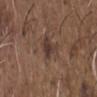follow-up: imaged on a skin check; not biopsied | subject: male, aged approximately 50 | lesion diameter: ~3 mm (longest diameter) | lighting: white-light illumination | image: 15 mm crop, total-body photography | automated metrics: a footprint of about 4.5 mm², an outline eccentricity of about 0.5 (0 = round, 1 = elongated), and a symmetry-axis asymmetry near 0.35; a border-irregularity index near 3.5/10, a color-variation rating of about 3.5/10, and radial color variation of about 1; a classifier nevus-likeness of about 5/100 and a lesion-detection confidence of about 100/100 | anatomic site: the chest.